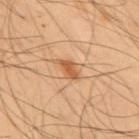Clinical impression: No biopsy was performed on this lesion — it was imaged during a full skin examination and was not determined to be concerning. Image and clinical context: Approximately 3 mm at its widest. Imaged with cross-polarized lighting. From the mid back. A 15 mm crop from a total-body photograph taken for skin-cancer surveillance. A male subject, aged around 55.